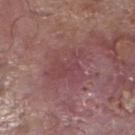Impression: The lesion was tiled from a total-body skin photograph and was not biopsied. Background: Located on the left lower leg. A 15 mm close-up extracted from a 3D total-body photography capture. The patient is a male aged around 80.